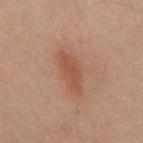Notes:
• workup · total-body-photography surveillance lesion; no biopsy
• patient · male, in their mid- to late 40s
• image · ~15 mm tile from a whole-body skin photo
• body site · the mid back